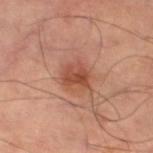Clinical impression: Part of a total-body skin-imaging series; this lesion was reviewed on a skin check and was not flagged for biopsy. Background: The lesion is located on the left thigh. The lesion's longest dimension is about 3.5 mm. The tile uses cross-polarized illumination. Cropped from a total-body skin-imaging series; the visible field is about 15 mm. The lesion-visualizer software estimated a nevus-likeness score of about 90/100.